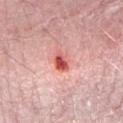Impression:
Recorded during total-body skin imaging; not selected for excision or biopsy.
Clinical summary:
A male patient aged approximately 50. The lesion's longest dimension is about 3 mm. A close-up tile cropped from a whole-body skin photograph, about 15 mm across. This is a white-light tile. An algorithmic analysis of the crop reported a border-irregularity rating of about 2.5/10 and internal color variation of about 6.5 on a 0–10 scale. Located on the right forearm.